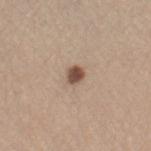workup=no biopsy performed (imaged during a skin exam) | lesion size=≈2 mm | patient=female, approximately 60 years of age | automated lesion analysis=an average lesion color of about L≈50 a*≈17 b*≈26 (CIELAB), roughly 15 lightness units darker than nearby skin, and a normalized border contrast of about 10.5; a classifier nevus-likeness of about 95/100 | image=total-body-photography crop, ~15 mm field of view | illumination=white-light illumination | body site=the right thigh.